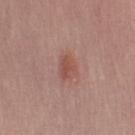Q: How was the tile lit?
A: white-light
Q: What is the lesion's diameter?
A: about 3 mm
Q: What are the patient's age and sex?
A: male, approximately 65 years of age
Q: Automated lesion metrics?
A: an area of roughly 4.5 mm², a shape eccentricity near 0.7, and a symmetry-axis asymmetry near 0.2; border irregularity of about 2 on a 0–10 scale, internal color variation of about 2 on a 0–10 scale, and radial color variation of about 0.5
Q: Lesion location?
A: the arm
Q: What is the imaging modality?
A: ~15 mm crop, total-body skin-cancer survey